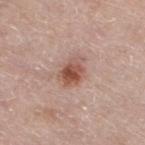{
  "site": "leg",
  "image": {
    "source": "total-body photography crop",
    "field_of_view_mm": 15
  },
  "lighting": "white-light",
  "patient": {
    "sex": "male",
    "age_approx": 65
  },
  "lesion_size": {
    "long_diameter_mm_approx": 4.0
  }
}